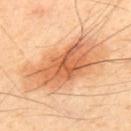{
  "biopsy_status": "not biopsied; imaged during a skin examination",
  "patient": {
    "sex": "male",
    "age_approx": 50
  },
  "image": {
    "source": "total-body photography crop",
    "field_of_view_mm": 15
  },
  "lesion_size": {
    "long_diameter_mm_approx": 10.5
  },
  "automated_metrics": {
    "area_mm2_approx": 40.0,
    "eccentricity": 0.9,
    "shape_asymmetry": 0.2,
    "border_irregularity_0_10": 3.5,
    "color_variation_0_10": 7.0,
    "peripheral_color_asymmetry": 2.0,
    "nevus_likeness_0_100": 95,
    "lesion_detection_confidence_0_100": 100
  },
  "site": "upper back",
  "lighting": "cross-polarized"
}